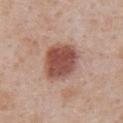Impression:
This lesion was catalogued during total-body skin photography and was not selected for biopsy.
Acquisition and patient details:
Located on the abdomen. This image is a 15 mm lesion crop taken from a total-body photograph. Captured under white-light illumination. The patient is a male in their mid- to late 60s.